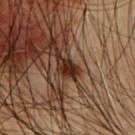This lesion was catalogued during total-body skin photography and was not selected for biopsy.
From the front of the torso.
A 15 mm crop from a total-body photograph taken for skin-cancer surveillance.
Imaged with cross-polarized lighting.
The patient is a male in their mid- to late 50s.
Approximately 3 mm at its widest.